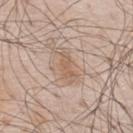* site — the mid back
* imaging modality — ~15 mm tile from a whole-body skin photo
* lighting — white-light
* patient — male, approximately 80 years of age
* lesion size — ≈4.5 mm